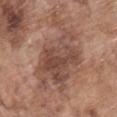Assessment:
The lesion was tiled from a total-body skin photograph and was not biopsied.
Clinical summary:
Located on the chest. A male subject, in their 70s. Cropped from a total-body skin-imaging series; the visible field is about 15 mm. This is a white-light tile.